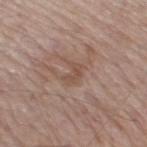Impression:
This lesion was catalogued during total-body skin photography and was not selected for biopsy.
Background:
The subject is a male in their 60s. Automated tile analysis of the lesion measured an area of roughly 3.5 mm², an eccentricity of roughly 0.7, and a shape-asymmetry score of about 0.4 (0 = symmetric). The software also gave a mean CIELAB color near L≈50 a*≈18 b*≈26, about 7 CIELAB-L* units darker than the surrounding skin, and a lesion-to-skin contrast of about 5.5 (normalized; higher = more distinct). It also reported border irregularity of about 4.5 on a 0–10 scale and a color-variation rating of about 1.5/10. The analysis additionally found an automated nevus-likeness rating near 0 out of 100. A close-up tile cropped from a whole-body skin photograph, about 15 mm across. This is a white-light tile. On the right thigh.